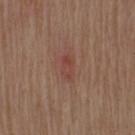The lesion was tiled from a total-body skin photograph and was not biopsied.
A 15 mm close-up extracted from a 3D total-body photography capture.
On the right upper arm.
Automated tile analysis of the lesion measured a lesion area of about 5 mm² and an eccentricity of roughly 0.85. And it measured a lesion–skin lightness drop of about 6 and a lesion-to-skin contrast of about 5 (normalized; higher = more distinct).
A male subject, in their 70s.
Measured at roughly 3.5 mm in maximum diameter.